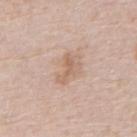biopsy_status: not biopsied; imaged during a skin examination
patient:
  sex: male
  age_approx: 80
image:
  source: total-body photography crop
  field_of_view_mm: 15
lesion_size:
  long_diameter_mm_approx: 3.5
site: abdomen
automated_metrics:
  area_mm2_approx: 5.0
  eccentricity: 0.85
  shape_asymmetry: 0.6
  vs_skin_darker_L: 8.0